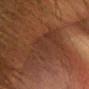Case summary:
– follow-up — catalogued during a skin exam; not biopsied
– illumination — cross-polarized
– TBP lesion metrics — a footprint of about 18 mm², an eccentricity of roughly 0.75, and a symmetry-axis asymmetry near 0.3; about 5 CIELAB-L* units darker than the surrounding skin and a normalized lesion–skin contrast near 5.5; a nevus-likeness score of about 5/100 and a detector confidence of about 60 out of 100 that the crop contains a lesion
– image source — total-body-photography crop, ~15 mm field of view
– site — the head or neck
– subject — female, approximately 65 years of age
– lesion diameter — about 6.5 mm